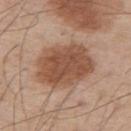Background:
A male subject, aged 53–57. From the upper back. This is a white-light tile. Automated image analysis of the tile measured an area of roughly 27 mm², an eccentricity of roughly 0.75, and a shape-asymmetry score of about 0.15 (0 = symmetric). The software also gave an average lesion color of about L≈50 a*≈21 b*≈30 (CIELAB). And it measured a classifier nevus-likeness of about 85/100 and a lesion-detection confidence of about 100/100. Longest diameter approximately 7 mm. A 15 mm close-up extracted from a 3D total-body photography capture.